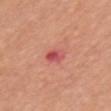Cropped from a total-body skin-imaging series; the visible field is about 15 mm.
About 2.5 mm across.
Captured under white-light illumination.
On the chest.
A female subject about 55 years old.